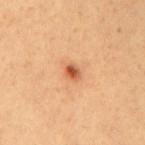Acquisition and patient details: The tile uses cross-polarized illumination. Automated image analysis of the tile measured a lesion color around L≈54 a*≈27 b*≈38 in CIELAB and roughly 14 lightness units darker than nearby skin. The lesion is on the back. Cropped from a total-body skin-imaging series; the visible field is about 15 mm. Measured at roughly 2 mm in maximum diameter. A male patient, aged 48–52.